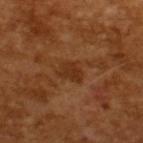<lesion>
  <biopsy_status>not biopsied; imaged during a skin examination</biopsy_status>
  <automated_metrics>
    <cielab_L>31</cielab_L>
    <cielab_a>22</cielab_a>
    <cielab_b>33</cielab_b>
    <vs_skin_darker_L>7.0</vs_skin_darker_L>
    <vs_skin_contrast_norm>7.0</vs_skin_contrast_norm>
    <border_irregularity_0_10>3.0</border_irregularity_0_10>
    <color_variation_0_10>1.5</color_variation_0_10>
    <peripheral_color_asymmetry>0.5</peripheral_color_asymmetry>
  </automated_metrics>
  <patient>
    <sex>male</sex>
    <age_approx>65</age_approx>
  </patient>
  <image>
    <source>total-body photography crop</source>
    <field_of_view_mm>15</field_of_view_mm>
  </image>
</lesion>